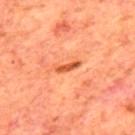Q: Was a biopsy performed?
A: total-body-photography surveillance lesion; no biopsy
Q: What did automated image analysis measure?
A: an area of roughly 3 mm², an outline eccentricity of about 0.95 (0 = round, 1 = elongated), and a shape-asymmetry score of about 0.35 (0 = symmetric); a lesion-detection confidence of about 100/100
Q: Lesion size?
A: about 3.5 mm
Q: Patient demographics?
A: male, aged 63–67
Q: What is the imaging modality?
A: ~15 mm tile from a whole-body skin photo
Q: Illumination type?
A: cross-polarized illumination
Q: Where on the body is the lesion?
A: the mid back